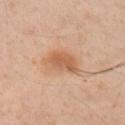Acquisition and patient details: This image is a 15 mm lesion crop taken from a total-body photograph. From the arm. A male subject approximately 45 years of age.